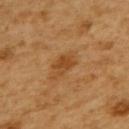Clinical impression:
The lesion was tiled from a total-body skin photograph and was not biopsied.
Acquisition and patient details:
Located on the upper back. This image is a 15 mm lesion crop taken from a total-body photograph. The patient is a female aged 53–57.